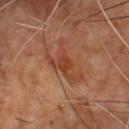– notes · total-body-photography surveillance lesion; no biopsy
– anatomic site · the chest
– imaging modality · 15 mm crop, total-body photography
– patient · male, roughly 55 years of age
– automated lesion analysis · a footprint of about 9.5 mm², an outline eccentricity of about 0.9 (0 = round, 1 = elongated), and a shape-asymmetry score of about 0.6 (0 = symmetric); an average lesion color of about L≈40 a*≈24 b*≈31 (CIELAB), a lesion–skin lightness drop of about 7, and a normalized lesion–skin contrast near 6.5; a color-variation rating of about 4/10 and a peripheral color-asymmetry measure near 1.5